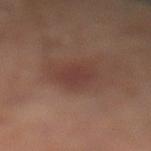  biopsy_status: not biopsied; imaged during a skin examination
  patient:
    sex: male
    age_approx: 65
  lesion_size:
    long_diameter_mm_approx: 3.0
  site: left lower leg
  image:
    source: total-body photography crop
    field_of_view_mm: 15
  lighting: cross-polarized